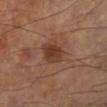notes=catalogued during a skin exam; not biopsied | image=~15 mm tile from a whole-body skin photo | body site=the left lower leg | patient=male, aged approximately 60 | diameter=~3.5 mm (longest diameter) | lighting=cross-polarized illumination.